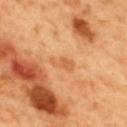Q: Is there a histopathology result?
A: imaged on a skin check; not biopsied
Q: Where on the body is the lesion?
A: the mid back
Q: Patient demographics?
A: male, approximately 50 years of age
Q: What kind of image is this?
A: ~15 mm tile from a whole-body skin photo
Q: What lighting was used for the tile?
A: cross-polarized illumination
Q: What did automated image analysis measure?
A: a footprint of about 4.5 mm²; about 7 CIELAB-L* units darker than the surrounding skin and a normalized border contrast of about 5; a peripheral color-asymmetry measure near 0.5; an automated nevus-likeness rating near 0 out of 100 and a lesion-detection confidence of about 100/100
Q: Lesion size?
A: ≈3.5 mm